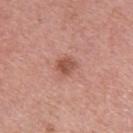This lesion was catalogued during total-body skin photography and was not selected for biopsy.
The total-body-photography lesion software estimated an average lesion color of about L≈53 a*≈25 b*≈28 (CIELAB) and a lesion–skin lightness drop of about 10. The analysis additionally found a border-irregularity index near 2/10 and peripheral color asymmetry of about 1. The analysis additionally found a detector confidence of about 100 out of 100 that the crop contains a lesion.
A roughly 15 mm field-of-view crop from a total-body skin photograph.
Captured under white-light illumination.
The patient is a female aged around 45.
Located on the left upper arm.
About 3 mm across.